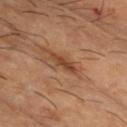Q: Was this lesion biopsied?
A: no biopsy performed (imaged during a skin exam)
Q: Lesion size?
A: ~3.5 mm (longest diameter)
Q: Automated lesion metrics?
A: a classifier nevus-likeness of about 5/100 and lesion-presence confidence of about 100/100
Q: Who is the patient?
A: male, aged 48–52
Q: How was the tile lit?
A: cross-polarized illumination
Q: What is the imaging modality?
A: ~15 mm crop, total-body skin-cancer survey
Q: Where on the body is the lesion?
A: the head or neck